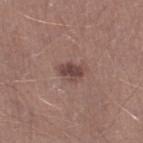workup: total-body-photography surveillance lesion; no biopsy | lighting: white-light | acquisition: ~15 mm tile from a whole-body skin photo | patient: male, aged 28–32 | lesion diameter: ≈3 mm.